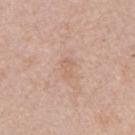biopsy_status: not biopsied; imaged during a skin examination
site: arm
patient:
  sex: female
  age_approx: 45
lighting: white-light
image:
  source: total-body photography crop
  field_of_view_mm: 15
automated_metrics:
  area_mm2_approx: 3.0
  eccentricity: 0.85
  shape_asymmetry: 0.45
  cielab_L: 63
  cielab_a: 19
  cielab_b: 30
  vs_skin_darker_L: 6.0
  vs_skin_contrast_norm: 4.5